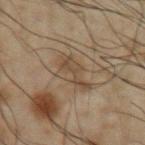Q: Was a biopsy performed?
A: catalogued during a skin exam; not biopsied
Q: What kind of image is this?
A: total-body-photography crop, ~15 mm field of view
Q: What is the lesion's diameter?
A: about 4 mm
Q: Patient demographics?
A: male, in their 50s
Q: What is the anatomic site?
A: the left upper arm
Q: Illumination type?
A: cross-polarized illumination